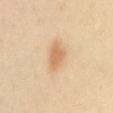biopsy_status: not biopsied; imaged during a skin examination
patient:
  sex: female
  age_approx: 40
lighting: cross-polarized
image:
  source: total-body photography crop
  field_of_view_mm: 15
site: mid back
lesion_size:
  long_diameter_mm_approx: 4.0